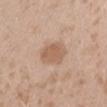| field | value |
|---|---|
| biopsy status | imaged on a skin check; not biopsied |
| diameter | ≈3.5 mm |
| imaging modality | total-body-photography crop, ~15 mm field of view |
| subject | female, aged around 25 |
| lighting | white-light |
| location | the right forearm |